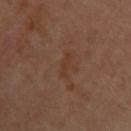Captured during whole-body skin photography for melanoma surveillance; the lesion was not biopsied. A female subject aged 58 to 62. The lesion is on the arm. Longest diameter approximately 3 mm. Cropped from a total-body skin-imaging series; the visible field is about 15 mm.